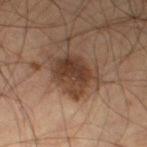Imaged during a routine full-body skin examination; the lesion was not biopsied and no histopathology is available. A male subject aged approximately 55. This image is a 15 mm lesion crop taken from a total-body photograph. The recorded lesion diameter is about 4.5 mm. The lesion is located on the left thigh. Captured under cross-polarized illumination. The lesion-visualizer software estimated a footprint of about 11 mm² and an outline eccentricity of about 0.65 (0 = round, 1 = elongated). The analysis additionally found a lesion color around L≈34 a*≈17 b*≈25 in CIELAB, about 12 CIELAB-L* units darker than the surrounding skin, and a lesion-to-skin contrast of about 10.5 (normalized; higher = more distinct). The software also gave border irregularity of about 4 on a 0–10 scale and a color-variation rating of about 4/10. It also reported an automated nevus-likeness rating near 50 out of 100 and a detector confidence of about 100 out of 100 that the crop contains a lesion.